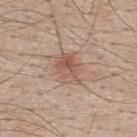Clinical impression: The lesion was tiled from a total-body skin photograph and was not biopsied. Background: A roughly 15 mm field-of-view crop from a total-body skin photograph. The lesion is on the back. Automated tile analysis of the lesion measured a mean CIELAB color near L≈56 a*≈20 b*≈27 and a normalized border contrast of about 6. The software also gave border irregularity of about 2.5 on a 0–10 scale, internal color variation of about 6 on a 0–10 scale, and radial color variation of about 2.5. The software also gave an automated nevus-likeness rating near 85 out of 100 and lesion-presence confidence of about 100/100. A male subject, about 50 years old. The recorded lesion diameter is about 4 mm.